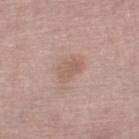No biopsy was performed on this lesion — it was imaged during a full skin examination and was not determined to be concerning. The lesion is located on the leg. The subject is a female aged around 70. A 15 mm close-up extracted from a 3D total-body photography capture.